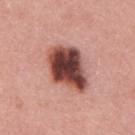{"biopsy_status": "not biopsied; imaged during a skin examination", "image": {"source": "total-body photography crop", "field_of_view_mm": 15}, "lesion_size": {"long_diameter_mm_approx": 6.5}, "site": "mid back", "patient": {"sex": "male", "age_approx": 60}, "lighting": "white-light"}